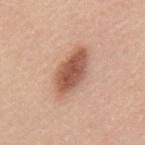Assessment:
Recorded during total-body skin imaging; not selected for excision or biopsy.
Image and clinical context:
The lesion is on the back. The patient is a male in their 40s. This is a white-light tile. A close-up tile cropped from a whole-body skin photograph, about 15 mm across. The recorded lesion diameter is about 6 mm.